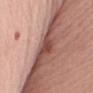The patient is a female roughly 65 years of age. From the right upper arm. A close-up tile cropped from a whole-body skin photograph, about 15 mm across.